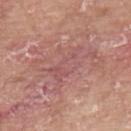{"biopsy_status": "not biopsied; imaged during a skin examination", "image": {"source": "total-body photography crop", "field_of_view_mm": 15}, "automated_metrics": {"area_mm2_approx": 25.0, "eccentricity": 0.85, "cielab_L": 55, "cielab_a": 24, "cielab_b": 23, "vs_skin_darker_L": 7.0, "vs_skin_contrast_norm": 5.0}, "patient": {"sex": "male", "age_approx": 65}, "site": "upper back", "lesion_size": {"long_diameter_mm_approx": 8.5}, "lighting": "white-light"}